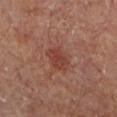<record>
<biopsy_status>not biopsied; imaged during a skin examination</biopsy_status>
<patient>
  <sex>male</sex>
  <age_approx>65</age_approx>
</patient>
<lighting>cross-polarized</lighting>
<site>left lower leg</site>
<image>
  <source>total-body photography crop</source>
  <field_of_view_mm>15</field_of_view_mm>
</image>
<automated_metrics>
  <color_variation_0_10>2.5</color_variation_0_10>
  <peripheral_color_asymmetry>1.0</peripheral_color_asymmetry>
  <lesion_detection_confidence_0_100>100</lesion_detection_confidence_0_100>
</automated_metrics>
<lesion_size>
  <long_diameter_mm_approx>3.0</long_diameter_mm_approx>
</lesion_size>
</record>